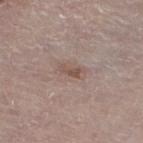workup: catalogued during a skin exam; not biopsied | location: the left lower leg | image-analysis metrics: an area of roughly 2.5 mm², an outline eccentricity of about 0.9 (0 = round, 1 = elongated), and two-axis asymmetry of about 0.4; a lesion-detection confidence of about 100/100 | tile lighting: white-light | diameter: about 2.5 mm | patient: male, aged 78 to 82 | imaging modality: total-body-photography crop, ~15 mm field of view.